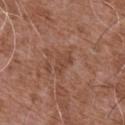Clinical impression:
No biopsy was performed on this lesion — it was imaged during a full skin examination and was not determined to be concerning.
Image and clinical context:
From the chest. A male patient, approximately 75 years of age. A roughly 15 mm field-of-view crop from a total-body skin photograph.